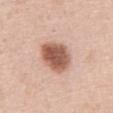  biopsy_status: not biopsied; imaged during a skin examination
  patient:
    sex: male
    age_approx: 50
  image:
    source: total-body photography crop
    field_of_view_mm: 15
  site: abdomen